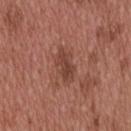biopsy status: imaged on a skin check; not biopsied | tile lighting: white-light | location: the head or neck | subject: male, aged 53 to 57 | image source: 15 mm crop, total-body photography | lesion size: ≈4 mm | automated lesion analysis: an outline eccentricity of about 0.85 (0 = round, 1 = elongated) and two-axis asymmetry of about 0.25; a classifier nevus-likeness of about 5/100.